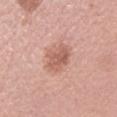Part of a total-body skin-imaging series; this lesion was reviewed on a skin check and was not flagged for biopsy.
This is a white-light tile.
Automated tile analysis of the lesion measured a peripheral color-asymmetry measure near 1. The analysis additionally found an automated nevus-likeness rating near 15 out of 100 and a lesion-detection confidence of about 100/100.
Located on the left upper arm.
The lesion's longest dimension is about 4 mm.
Cropped from a whole-body photographic skin survey; the tile spans about 15 mm.
The subject is a female aged 48–52.